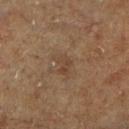No biopsy was performed on this lesion — it was imaged during a full skin examination and was not determined to be concerning. This image is a 15 mm lesion crop taken from a total-body photograph. The lesion is located on the right lower leg. The subject is a male approximately 75 years of age.